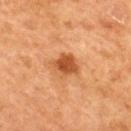| key | value |
|---|---|
| biopsy status | catalogued during a skin exam; not biopsied |
| diameter | ≈3.5 mm |
| imaging modality | ~15 mm crop, total-body skin-cancer survey |
| subject | female, approximately 40 years of age |
| location | the mid back |
| illumination | cross-polarized |
| image-analysis metrics | a lesion area of about 7.5 mm², a shape eccentricity near 0.65, and a shape-asymmetry score of about 0.25 (0 = symmetric); a border-irregularity index near 3/10, a within-lesion color-variation index near 3/10, and a peripheral color-asymmetry measure near 1; a nevus-likeness score of about 85/100 and a detector confidence of about 100 out of 100 that the crop contains a lesion |